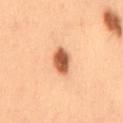  biopsy_status: not biopsied; imaged during a skin examination
  image:
    source: total-body photography crop
    field_of_view_mm: 15
  lesion_size:
    long_diameter_mm_approx: 3.5
  site: lower back
  patient:
    sex: male
    age_approx: 55
  automated_metrics:
    cielab_L: 47
    cielab_a: 23
    cielab_b: 32
    vs_skin_darker_L: 16.0
    vs_skin_contrast_norm: 11.5
    color_variation_0_10: 3.5
    peripheral_color_asymmetry: 1.0
    nevus_likeness_0_100: 100
    lesion_detection_confidence_0_100: 100